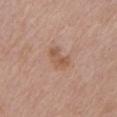This lesion was catalogued during total-body skin photography and was not selected for biopsy.
The lesion-visualizer software estimated an area of roughly 5.5 mm², an outline eccentricity of about 0.8 (0 = round, 1 = elongated), and a shape-asymmetry score of about 0.3 (0 = symmetric). The analysis additionally found about 8 CIELAB-L* units darker than the surrounding skin. The analysis additionally found a within-lesion color-variation index near 3/10.
The tile uses white-light illumination.
Located on the left upper arm.
The patient is a male aged 73–77.
The lesion's longest dimension is about 3.5 mm.
A 15 mm crop from a total-body photograph taken for skin-cancer surveillance.